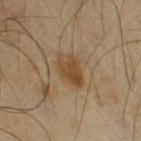biopsy_status: not biopsied; imaged during a skin examination
image:
  source: total-body photography crop
  field_of_view_mm: 15
lighting: cross-polarized
lesion_size:
  long_diameter_mm_approx: 4.0
site: right upper arm
patient:
  sex: male
  age_approx: 65